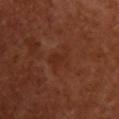Assessment: Part of a total-body skin-imaging series; this lesion was reviewed on a skin check and was not flagged for biopsy. Clinical summary: The tile uses cross-polarized illumination. A 15 mm close-up tile from a total-body photography series done for melanoma screening. The lesion-visualizer software estimated a footprint of about 5.5 mm² and two-axis asymmetry of about 0.4. And it measured a lesion color around L≈28 a*≈23 b*≈28 in CIELAB, roughly 4 lightness units darker than nearby skin, and a normalized lesion–skin contrast near 4.5. It also reported a border-irregularity index near 5/10, a color-variation rating of about 1.5/10, and radial color variation of about 0.5. A female patient aged approximately 65. The lesion is on the chest. The recorded lesion diameter is about 3.5 mm.